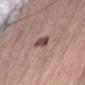Notes:
- notes: imaged on a skin check; not biopsied
- anatomic site: the lower back
- illumination: white-light
- image-analysis metrics: a within-lesion color-variation index near 3/10 and peripheral color asymmetry of about 1
- diameter: ~3 mm (longest diameter)
- subject: male, in their mid-60s
- imaging modality: 15 mm crop, total-body photography